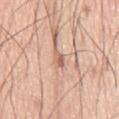Impression:
Captured during whole-body skin photography for melanoma surveillance; the lesion was not biopsied.
Context:
On the chest. Imaged with white-light lighting. Cropped from a whole-body photographic skin survey; the tile spans about 15 mm. The subject is a male aged 73–77.